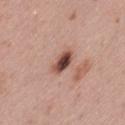Imaged during a routine full-body skin examination; the lesion was not biopsied and no histopathology is available. A female subject aged 28–32. The recorded lesion diameter is about 3.5 mm. An algorithmic analysis of the crop reported a footprint of about 5.5 mm² and an eccentricity of roughly 0.8. It also reported a within-lesion color-variation index near 9/10 and peripheral color asymmetry of about 2.5. A roughly 15 mm field-of-view crop from a total-body skin photograph. Located on the left thigh. The tile uses white-light illumination.